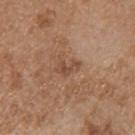follow-up = total-body-photography surveillance lesion; no biopsy
subject = male, roughly 70 years of age
image-analysis metrics = an area of roughly 4 mm² and a shape eccentricity near 0.85; a normalized border contrast of about 6
body site = the chest
lighting = white-light
image source = ~15 mm tile from a whole-body skin photo
size = ~3 mm (longest diameter)